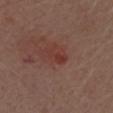| field | value |
|---|---|
| notes | total-body-photography surveillance lesion; no biopsy |
| acquisition | ~15 mm tile from a whole-body skin photo |
| body site | the right upper arm |
| subject | male, about 70 years old |
| lesion size | about 3 mm |